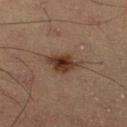The subject is a male aged approximately 60. A 15 mm close-up extracted from a 3D total-body photography capture. From the right lower leg. The tile uses cross-polarized illumination. The recorded lesion diameter is about 4 mm.